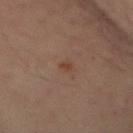The lesion was tiled from a total-body skin photograph and was not biopsied.
A male subject, aged 63–67.
The tile uses cross-polarized illumination.
From the mid back.
A lesion tile, about 15 mm wide, cut from a 3D total-body photograph.